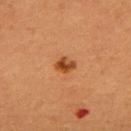follow-up: total-body-photography surveillance lesion; no biopsy | lesion size: about 2.5 mm | image source: total-body-photography crop, ~15 mm field of view | subject: male, roughly 40 years of age | anatomic site: the back | tile lighting: cross-polarized illumination.